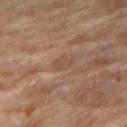workup=imaged on a skin check; not biopsied
patient=female, approximately 70 years of age
illumination=cross-polarized
location=the right thigh
image source=15 mm crop, total-body photography